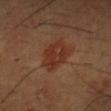Q: Patient demographics?
A: male, aged 43 to 47
Q: What is the lesion's diameter?
A: ≈5 mm
Q: What is the imaging modality?
A: ~15 mm tile from a whole-body skin photo
Q: What lighting was used for the tile?
A: cross-polarized illumination
Q: Lesion location?
A: the chest
Q: What did automated image analysis measure?
A: a lesion color around L≈32 a*≈22 b*≈29 in CIELAB, a lesion–skin lightness drop of about 7, and a normalized lesion–skin contrast near 7; border irregularity of about 3 on a 0–10 scale, a color-variation rating of about 3/10, and a peripheral color-asymmetry measure near 1; a classifier nevus-likeness of about 70/100